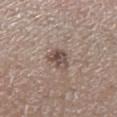The lesion was photographed on a routine skin check and not biopsied; there is no pathology result. A female subject aged 68 to 72. Located on the left lower leg. The lesion-visualizer software estimated an area of roughly 5.5 mm² and a shape-asymmetry score of about 0.35 (0 = symmetric). The analysis additionally found a mean CIELAB color near L≈49 a*≈14 b*≈21, roughly 11 lightness units darker than nearby skin, and a normalized lesion–skin contrast near 8.5. And it measured an automated nevus-likeness rating near 10 out of 100 and lesion-presence confidence of about 100/100. This image is a 15 mm lesion crop taken from a total-body photograph. Imaged with white-light lighting. Measured at roughly 3 mm in maximum diameter.